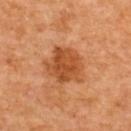<case>
<biopsy_status>not biopsied; imaged during a skin examination</biopsy_status>
<site>upper back</site>
<patient>
  <sex>female</sex>
  <age_approx>50</age_approx>
</patient>
<lesion_size>
  <long_diameter_mm_approx>5.0</long_diameter_mm_approx>
</lesion_size>
<automated_metrics>
  <vs_skin_darker_L>11.0</vs_skin_darker_L>
  <vs_skin_contrast_norm>8.0</vs_skin_contrast_norm>
  <nevus_likeness_0_100>75</nevus_likeness_0_100>
  <lesion_detection_confidence_0_100>100</lesion_detection_confidence_0_100>
</automated_metrics>
<lighting>cross-polarized</lighting>
<image>
  <source>total-body photography crop</source>
  <field_of_view_mm>15</field_of_view_mm>
</image>
</case>